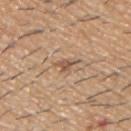biopsy_status: not biopsied; imaged during a skin examination
site: arm
lesion_size:
  long_diameter_mm_approx: 2.5
automated_metrics:
  area_mm2_approx: 3.0
  eccentricity: 0.75
  shape_asymmetry: 0.45
lighting: white-light
image:
  source: total-body photography crop
  field_of_view_mm: 15
patient:
  sex: male
  age_approx: 60